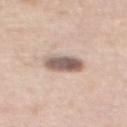biopsy_status: not biopsied; imaged during a skin examination
automated_metrics:
  border_irregularity_0_10: 2.0
  color_variation_0_10: 5.0
  peripheral_color_asymmetry: 1.5
image:
  source: total-body photography crop
  field_of_view_mm: 15
lighting: white-light
site: back
patient:
  sex: female
  age_approx: 65